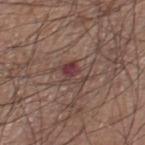Captured during whole-body skin photography for melanoma surveillance; the lesion was not biopsied. A roughly 15 mm field-of-view crop from a total-body skin photograph. From the leg. Imaged with white-light lighting. Automated tile analysis of the lesion measured a mean CIELAB color near L≈39 a*≈21 b*≈20, a lesion–skin lightness drop of about 9, and a normalized lesion–skin contrast near 8. And it measured peripheral color asymmetry of about 1.5. And it measured a classifier nevus-likeness of about 10/100 and a detector confidence of about 100 out of 100 that the crop contains a lesion. The lesion's longest dimension is about 3 mm. The patient is a male approximately 60 years of age.